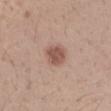The lesion was photographed on a routine skin check and not biopsied; there is no pathology result.
The total-body-photography lesion software estimated a lesion area of about 5.5 mm², a shape eccentricity near 0.2, and a shape-asymmetry score of about 0.15 (0 = symmetric). The analysis additionally found a lesion-detection confidence of about 100/100.
About 2.5 mm across.
This is a white-light tile.
On the left forearm.
A male patient about 30 years old.
A roughly 15 mm field-of-view crop from a total-body skin photograph.